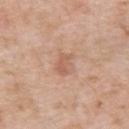subject: male, about 60 years old
location: the upper back
automated metrics: a lesion-detection confidence of about 100/100
image: ~15 mm crop, total-body skin-cancer survey
lighting: white-light
size: ≈2.5 mm